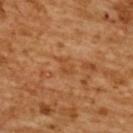Captured during whole-body skin photography for melanoma surveillance; the lesion was not biopsied. Imaged with cross-polarized lighting. A 15 mm crop from a total-body photograph taken for skin-cancer surveillance. The lesion is on the upper back. The lesion's longest dimension is about 2.5 mm. An algorithmic analysis of the crop reported a lesion-detection confidence of about 100/100. A female patient aged around 55.